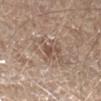Q: Was a biopsy performed?
A: no biopsy performed (imaged during a skin exam)
Q: How was the tile lit?
A: white-light
Q: What are the patient's age and sex?
A: male, aged approximately 70
Q: Lesion location?
A: the left forearm
Q: Automated lesion metrics?
A: an area of roughly 3 mm², an eccentricity of roughly 0.9, and a symmetry-axis asymmetry near 0.55; internal color variation of about 1.5 on a 0–10 scale and peripheral color asymmetry of about 0.5
Q: What is the imaging modality?
A: ~15 mm tile from a whole-body skin photo
Q: How large is the lesion?
A: ≈3 mm